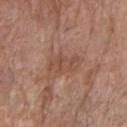Imaged during a routine full-body skin examination; the lesion was not biopsied and no histopathology is available. A close-up tile cropped from a whole-body skin photograph, about 15 mm across. Automated tile analysis of the lesion measured a lesion area of about 6.5 mm². The analysis additionally found a lesion color around L≈49 a*≈21 b*≈28 in CIELAB, roughly 7 lightness units darker than nearby skin, and a normalized lesion–skin contrast near 5. And it measured a lesion-detection confidence of about 100/100. Imaged with white-light lighting. From the right forearm. Measured at roughly 3.5 mm in maximum diameter. The patient is a female roughly 75 years of age.